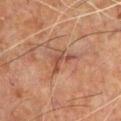Q: Was this lesion biopsied?
A: imaged on a skin check; not biopsied
Q: What is the anatomic site?
A: the chest
Q: What kind of image is this?
A: ~15 mm tile from a whole-body skin photo
Q: What is the lesion's diameter?
A: ≈3 mm
Q: Who is the patient?
A: male, about 50 years old
Q: Illumination type?
A: cross-polarized